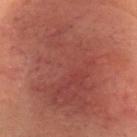Part of a total-body skin-imaging series; this lesion was reviewed on a skin check and was not flagged for biopsy. A female subject about 40 years old. This is a cross-polarized tile. Approximately 17 mm at its widest. A region of skin cropped from a whole-body photographic capture, roughly 15 mm wide. On the head or neck.